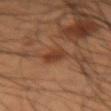The subject is a male aged 33–37. This is a cross-polarized tile. Located on the right forearm. This image is a 15 mm lesion crop taken from a total-body photograph. About 2.5 mm across.